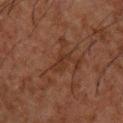{
  "biopsy_status": "not biopsied; imaged during a skin examination",
  "image": {
    "source": "total-body photography crop",
    "field_of_view_mm": 15
  },
  "lighting": "cross-polarized",
  "site": "chest",
  "patient": {
    "sex": "male",
    "age_approx": 50
  },
  "lesion_size": {
    "long_diameter_mm_approx": 2.5
  },
  "automated_metrics": {
    "area_mm2_approx": 2.5,
    "eccentricity": 0.9,
    "shape_asymmetry": 0.4,
    "peripheral_color_asymmetry": 0.0
  }
}